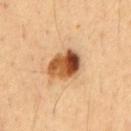biopsy_status: not biopsied; imaged during a skin examination
lesion_size:
  long_diameter_mm_approx: 4.5
site: front of the torso
patient:
  sex: male
  age_approx: 55
image:
  source: total-body photography crop
  field_of_view_mm: 15
lighting: cross-polarized